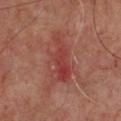Notes:
– biopsy status: catalogued during a skin exam; not biopsied
– image source: ~15 mm crop, total-body skin-cancer survey
– automated lesion analysis: a lesion–skin lightness drop of about 8 and a normalized border contrast of about 6.5; a border-irregularity rating of about 5.5/10, a color-variation rating of about 4.5/10, and peripheral color asymmetry of about 1.5; a nevus-likeness score of about 0/100
– patient: approximately 55 years of age
– anatomic site: the front of the torso
– lighting: cross-polarized
– size: ≈6 mm